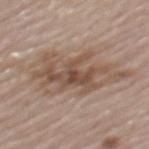| feature | finding |
|---|---|
| notes | no biopsy performed (imaged during a skin exam) |
| lighting | white-light |
| automated lesion analysis | border irregularity of about 8 on a 0–10 scale and a within-lesion color-variation index near 7/10 |
| subject | male, aged approximately 80 |
| location | the mid back |
| image source | ~15 mm crop, total-body skin-cancer survey |